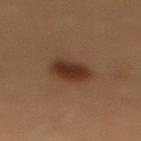{"biopsy_status": "not biopsied; imaged during a skin examination", "site": "upper back", "lighting": "cross-polarized", "patient": {"sex": "female", "age_approx": 50}, "image": {"source": "total-body photography crop", "field_of_view_mm": 15}}